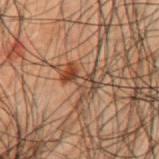Q: Is there a histopathology result?
A: total-body-photography surveillance lesion; no biopsy
Q: What are the patient's age and sex?
A: male, roughly 50 years of age
Q: How large is the lesion?
A: about 4 mm
Q: What is the anatomic site?
A: the mid back
Q: What did automated image analysis measure?
A: an area of roughly 7 mm², an eccentricity of roughly 0.9, and two-axis asymmetry of about 0.35; roughly 9 lightness units darker than nearby skin and a normalized lesion–skin contrast near 8; a nevus-likeness score of about 25/100 and lesion-presence confidence of about 100/100
Q: How was this image acquired?
A: total-body-photography crop, ~15 mm field of view
Q: How was the tile lit?
A: cross-polarized illumination